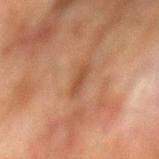On the back.
The recorded lesion diameter is about 2.5 mm.
Imaged with cross-polarized lighting.
A 15 mm crop from a total-body photograph taken for skin-cancer surveillance.
The total-body-photography lesion software estimated a mean CIELAB color near L≈41 a*≈20 b*≈30 and about 7 CIELAB-L* units darker than the surrounding skin. And it measured a peripheral color-asymmetry measure near 0. And it measured a nevus-likeness score of about 0/100 and a lesion-detection confidence of about 100/100.
A male subject, aged approximately 75.